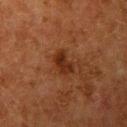Case summary:
- biopsy status: total-body-photography surveillance lesion; no biopsy
- lighting: cross-polarized
- image source: ~15 mm tile from a whole-body skin photo
- location: the left upper arm
- lesion diameter: about 3 mm
- image-analysis metrics: a lesion area of about 4.5 mm², an outline eccentricity of about 0.8 (0 = round, 1 = elongated), and a shape-asymmetry score of about 0.25 (0 = symmetric); a lesion color around L≈24 a*≈21 b*≈27 in CIELAB, about 8 CIELAB-L* units darker than the surrounding skin, and a normalized border contrast of about 9; a color-variation rating of about 2.5/10 and radial color variation of about 1; a classifier nevus-likeness of about 25/100 and a detector confidence of about 100 out of 100 that the crop contains a lesion
- patient: female, approximately 50 years of age